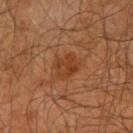Q: Was a biopsy performed?
A: imaged on a skin check; not biopsied
Q: What kind of image is this?
A: ~15 mm tile from a whole-body skin photo
Q: What is the anatomic site?
A: the arm
Q: Who is the patient?
A: male, aged around 65